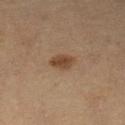Assessment:
Part of a total-body skin-imaging series; this lesion was reviewed on a skin check and was not flagged for biopsy.
Acquisition and patient details:
A patient about 60 years old. Approximately 3 mm at its widest. The lesion is on the leg. A close-up tile cropped from a whole-body skin photograph, about 15 mm across. Imaged with cross-polarized lighting.